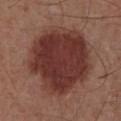Q: Was a biopsy performed?
A: no biopsy performed (imaged during a skin exam)
Q: How was this image acquired?
A: total-body-photography crop, ~15 mm field of view
Q: What is the lesion's diameter?
A: ≈8.5 mm
Q: What did automated image analysis measure?
A: a lesion area of about 55 mm²; a lesion color around L≈35 a*≈23 b*≈23 in CIELAB, about 13 CIELAB-L* units darker than the surrounding skin, and a normalized border contrast of about 11; a border-irregularity rating of about 1.5/10 and radial color variation of about 1; an automated nevus-likeness rating near 100 out of 100
Q: What are the patient's age and sex?
A: male, roughly 55 years of age
Q: Where on the body is the lesion?
A: the abdomen